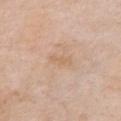The lesion was photographed on a routine skin check and not biopsied; there is no pathology result.
On the chest.
A close-up tile cropped from a whole-body skin photograph, about 15 mm across.
A female subject, aged approximately 75.